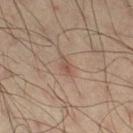Clinical impression: The lesion was tiled from a total-body skin photograph and was not biopsied. Context: Longest diameter approximately 2.5 mm. An algorithmic analysis of the crop reported an eccentricity of roughly 0.85 and two-axis asymmetry of about 0.3. A roughly 15 mm field-of-view crop from a total-body skin photograph. A male subject roughly 35 years of age. The lesion is on the right thigh.